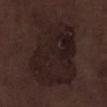<lesion>
  <biopsy_status>not biopsied; imaged during a skin examination</biopsy_status>
  <site>leg</site>
  <patient>
    <sex>male</sex>
    <age_approx>70</age_approx>
  </patient>
  <lighting>white-light</lighting>
  <automated_metrics>
    <border_irregularity_0_10>3.0</border_irregularity_0_10>
    <color_variation_0_10>4.0</color_variation_0_10>
    <peripheral_color_asymmetry>1.0</peripheral_color_asymmetry>
    <nevus_likeness_0_100>0</nevus_likeness_0_100>
    <lesion_detection_confidence_0_100>90</lesion_detection_confidence_0_100>
  </automated_metrics>
  <image>
    <source>total-body photography crop</source>
    <field_of_view_mm>15</field_of_view_mm>
  </image>
</lesion>